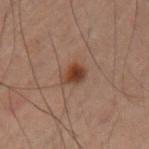workup: imaged on a skin check; not biopsied
acquisition: ~15 mm crop, total-body skin-cancer survey
subject: male, about 60 years old
body site: the left upper arm
illumination: cross-polarized